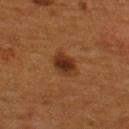Clinical impression:
Captured during whole-body skin photography for melanoma surveillance; the lesion was not biopsied.
Acquisition and patient details:
A roughly 15 mm field-of-view crop from a total-body skin photograph. The subject is a male aged 53 to 57. An algorithmic analysis of the crop reported a footprint of about 6 mm². It also reported a nevus-likeness score of about 100/100 and lesion-presence confidence of about 100/100. The lesion's longest dimension is about 3.5 mm. The lesion is on the upper back.